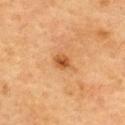Clinical impression: Recorded during total-body skin imaging; not selected for excision or biopsy. Acquisition and patient details: A 15 mm crop from a total-body photograph taken for skin-cancer surveillance. The subject is a female aged 58 to 62. Approximately 2.5 mm at its widest. The lesion is on the upper back.